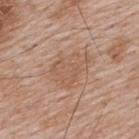This lesion was catalogued during total-body skin photography and was not selected for biopsy.
The tile uses white-light illumination.
A male patient in their mid- to late 60s.
The lesion-visualizer software estimated an area of roughly 13 mm² and a shape-asymmetry score of about 0.35 (0 = symmetric). It also reported a lesion color around L≈57 a*≈18 b*≈30 in CIELAB, a lesion–skin lightness drop of about 6, and a normalized lesion–skin contrast near 5. The software also gave border irregularity of about 4 on a 0–10 scale, a color-variation rating of about 2.5/10, and a peripheral color-asymmetry measure near 1.
This image is a 15 mm lesion crop taken from a total-body photograph.
The recorded lesion diameter is about 5 mm.
Located on the upper back.